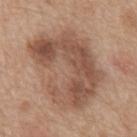automated lesion analysis — a footprint of about 41 mm²; an average lesion color of about L≈51 a*≈19 b*≈28 (CIELAB) and a lesion-to-skin contrast of about 8 (normalized; higher = more distinct) | acquisition — total-body-photography crop, ~15 mm field of view | body site — the abdomen | subject — male, aged approximately 65 | lesion diameter — ~9.5 mm (longest diameter).